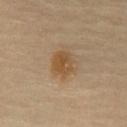acquisition=~15 mm crop, total-body skin-cancer survey
anatomic site=the chest
lesion size=~3.5 mm (longest diameter)
automated metrics=border irregularity of about 2.5 on a 0–10 scale, a color-variation rating of about 3.5/10, and a peripheral color-asymmetry measure near 1; a nevus-likeness score of about 90/100 and a detector confidence of about 100 out of 100 that the crop contains a lesion
subject=male, aged 68 to 72
illumination=cross-polarized illumination